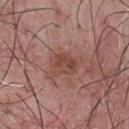biopsy status=total-body-photography surveillance lesion; no biopsy
acquisition=total-body-photography crop, ~15 mm field of view
tile lighting=white-light illumination
patient=male, aged 53 to 57
site=the front of the torso
size=≈4.5 mm